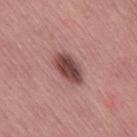The lesion was tiled from a total-body skin photograph and was not biopsied. A female subject about 50 years old. This is a white-light tile. The lesion's longest dimension is about 4.5 mm. Located on the leg. Cropped from a total-body skin-imaging series; the visible field is about 15 mm.